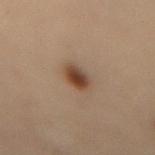lesion size: ≈3.5 mm | location: the lower back | lighting: cross-polarized | subject: female, about 30 years old | image: total-body-photography crop, ~15 mm field of view.